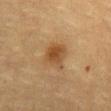biopsy_status: not biopsied; imaged during a skin examination
site: front of the torso
image:
  source: total-body photography crop
  field_of_view_mm: 15
patient:
  sex: female
  age_approx: 55